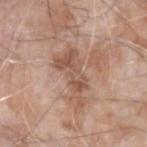lesion_size:
  long_diameter_mm_approx: 6.0
site: left forearm
image:
  source: total-body photography crop
  field_of_view_mm: 15
lighting: white-light
patient:
  sex: male
  age_approx: 75